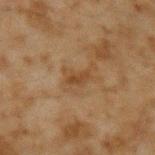Assessment: No biopsy was performed on this lesion — it was imaged during a full skin examination and was not determined to be concerning. Image and clinical context: On the left upper arm. The recorded lesion diameter is about 3 mm. Cropped from a total-body skin-imaging series; the visible field is about 15 mm. The tile uses cross-polarized illumination. The patient is a male aged 43 to 47.